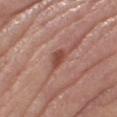notes: total-body-photography surveillance lesion; no biopsy | lighting: white-light illumination | image-analysis metrics: a lesion area of about 3 mm² and a shape eccentricity near 0.85; a lesion color around L≈46 a*≈25 b*≈27 in CIELAB and a lesion-to-skin contrast of about 8.5 (normalized; higher = more distinct); a border-irregularity rating of about 3.5/10, a color-variation rating of about 1/10, and a peripheral color-asymmetry measure near 0; a detector confidence of about 100 out of 100 that the crop contains a lesion | anatomic site: the right thigh | imaging modality: 15 mm crop, total-body photography | patient: female, in their mid-60s.